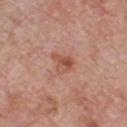biopsy status = catalogued during a skin exam; not biopsied
lighting = white-light illumination
anatomic site = the chest
image = 15 mm crop, total-body photography
patient = male, aged approximately 60